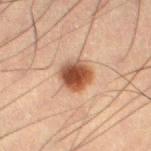follow-up = catalogued during a skin exam; not biopsied | subject = male, roughly 55 years of age | body site = the right thigh | acquisition = 15 mm crop, total-body photography | lighting = cross-polarized.